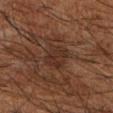Part of a total-body skin-imaging series; this lesion was reviewed on a skin check and was not flagged for biopsy.
An algorithmic analysis of the crop reported an area of roughly 8 mm², an eccentricity of roughly 0.8, and a shape-asymmetry score of about 0.2 (0 = symmetric). The analysis additionally found a nevus-likeness score of about 0/100.
Longest diameter approximately 4 mm.
The subject is a male aged 63–67.
On the right forearm.
A 15 mm crop from a total-body photograph taken for skin-cancer surveillance.
Captured under cross-polarized illumination.